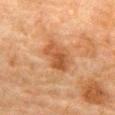– TBP lesion metrics: a symmetry-axis asymmetry near 0.3; peripheral color asymmetry of about 1.5; an automated nevus-likeness rating near 20 out of 100
– tile lighting: cross-polarized
– acquisition: ~15 mm tile from a whole-body skin photo
– diameter: about 4 mm
– body site: the abdomen
– patient: male, approximately 80 years of age